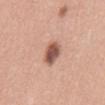This lesion was catalogued during total-body skin photography and was not selected for biopsy.
From the mid back.
An algorithmic analysis of the crop reported an average lesion color of about L≈55 a*≈22 b*≈27 (CIELAB) and roughly 16 lightness units darker than nearby skin. The analysis additionally found border irregularity of about 2 on a 0–10 scale, a within-lesion color-variation index near 4.5/10, and radial color variation of about 1.5.
A female patient, approximately 45 years of age.
This is a white-light tile.
A close-up tile cropped from a whole-body skin photograph, about 15 mm across.
The lesion's longest dimension is about 4 mm.